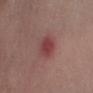  biopsy_status: not biopsied; imaged during a skin examination
  patient:
    sex: male
    age_approx: 70
  automated_metrics:
    area_mm2_approx: 6.0
    eccentricity: 0.55
    shape_asymmetry: 0.15
    cielab_L: 41
    cielab_a: 27
    cielab_b: 20
    vs_skin_darker_L: 9.0
    vs_skin_contrast_norm: 7.5
    nevus_likeness_0_100: 0
    lesion_detection_confidence_0_100: 100
  image:
    source: total-body photography crop
    field_of_view_mm: 15
  lesion_size:
    long_diameter_mm_approx: 3.0
  site: left lower leg